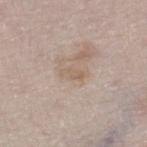Findings:
* biopsy status · catalogued during a skin exam; not biopsied
* size · about 2.5 mm
* location · the right lower leg
* image source · 15 mm crop, total-body photography
* subject · female, approximately 60 years of age